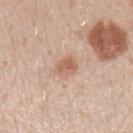| key | value |
|---|---|
| workup | catalogued during a skin exam; not biopsied |
| size | about 3 mm |
| imaging modality | total-body-photography crop, ~15 mm field of view |
| patient | male, aged approximately 30 |
| image-analysis metrics | a mean CIELAB color near L≈61 a*≈20 b*≈30; a classifier nevus-likeness of about 80/100 |
| anatomic site | the left forearm |
| illumination | white-light |